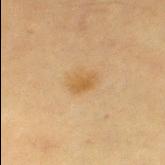Notes:
– follow-up — total-body-photography surveillance lesion; no biopsy
– imaging modality — total-body-photography crop, ~15 mm field of view
– tile lighting — cross-polarized
– anatomic site — the left thigh
– patient — female, aged around 55
– size — ≈3 mm